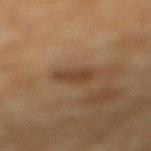Clinical summary:
Automated tile analysis of the lesion measured an area of roughly 6 mm², an outline eccentricity of about 0.8 (0 = round, 1 = elongated), and a symmetry-axis asymmetry near 0.2. The software also gave an automated nevus-likeness rating near 15 out of 100 and lesion-presence confidence of about 100/100. The patient is a male in their 60s. This image is a 15 mm lesion crop taken from a total-body photograph. Longest diameter approximately 3.5 mm. The lesion is on the right lower leg. This is a cross-polarized tile.